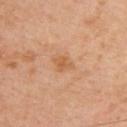{"biopsy_status": "not biopsied; imaged during a skin examination"}